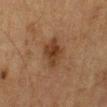notes: catalogued during a skin exam; not biopsied | image-analysis metrics: a lesion area of about 7.5 mm², an outline eccentricity of about 0.75 (0 = round, 1 = elongated), and a shape-asymmetry score of about 0.25 (0 = symmetric); a color-variation rating of about 2.5/10 and radial color variation of about 0.5; an automated nevus-likeness rating near 85 out of 100 and lesion-presence confidence of about 100/100 | anatomic site: the left lower leg | illumination: cross-polarized illumination | lesion diameter: ≈3.5 mm | image source: total-body-photography crop, ~15 mm field of view | patient: male, in their mid-80s or older.